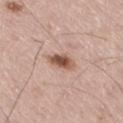Findings:
– workup · no biopsy performed (imaged during a skin exam)
– subject · male, roughly 55 years of age
– lesion size · ≈3 mm
– body site · the right thigh
– image-analysis metrics · a lesion–skin lightness drop of about 14 and a lesion-to-skin contrast of about 9.5 (normalized; higher = more distinct)
– acquisition · ~15 mm crop, total-body skin-cancer survey
– tile lighting · white-light illumination